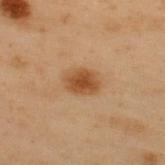<tbp_lesion>
  <biopsy_status>not biopsied; imaged during a skin examination</biopsy_status>
  <patient>
    <sex>male</sex>
    <age_approx>55</age_approx>
  </patient>
  <automated_metrics>
    <area_mm2_approx>7.5</area_mm2_approx>
    <eccentricity>0.7</eccentricity>
    <shape_asymmetry>0.15</shape_asymmetry>
    <color_variation_0_10>2.5</color_variation_0_10>
    <peripheral_color_asymmetry>0.5</peripheral_color_asymmetry>
    <nevus_likeness_0_100>95</nevus_likeness_0_100>
    <lesion_detection_confidence_0_100>100</lesion_detection_confidence_0_100>
  </automated_metrics>
  <lighting>cross-polarized</lighting>
  <image>
    <source>total-body photography crop</source>
    <field_of_view_mm>15</field_of_view_mm>
  </image>
  <site>upper back</site>
</tbp_lesion>